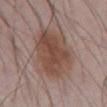No biopsy was performed on this lesion — it was imaged during a full skin examination and was not determined to be concerning. From the abdomen. This is a white-light tile. About 7 mm across. A male patient approximately 70 years of age. This image is a 15 mm lesion crop taken from a total-body photograph.